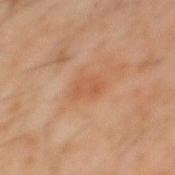Imaged during a routine full-body skin examination; the lesion was not biopsied and no histopathology is available. An algorithmic analysis of the crop reported a lesion area of about 4.5 mm², a shape eccentricity near 0.8, and a shape-asymmetry score of about 0.3 (0 = symmetric). The software also gave a mean CIELAB color near L≈51 a*≈22 b*≈34, about 6 CIELAB-L* units darker than the surrounding skin, and a normalized lesion–skin contrast near 5. A close-up tile cropped from a whole-body skin photograph, about 15 mm across. Imaged with cross-polarized lighting. On the back. The lesion's longest dimension is about 3 mm. A male patient approximately 60 years of age.